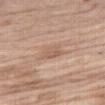<case>
<biopsy_status>not biopsied; imaged during a skin examination</biopsy_status>
<image>
  <source>total-body photography crop</source>
  <field_of_view_mm>15</field_of_view_mm>
</image>
<lighting>white-light</lighting>
<site>right thigh</site>
<automated_metrics>
  <area_mm2_approx>4.5</area_mm2_approx>
  <eccentricity>0.6</eccentricity>
  <shape_asymmetry>0.25</shape_asymmetry>
  <cielab_L>59</cielab_L>
  <cielab_a>19</cielab_a>
  <cielab_b>29</cielab_b>
  <vs_skin_darker_L>7.0</vs_skin_darker_L>
  <vs_skin_contrast_norm>5.0</vs_skin_contrast_norm>
  <border_irregularity_0_10>2.0</border_irregularity_0_10>
  <color_variation_0_10>2.0</color_variation_0_10>
  <peripheral_color_asymmetry>0.5</peripheral_color_asymmetry>
</automated_metrics>
<patient>
  <sex>male</sex>
  <age_approx>70</age_approx>
</patient>
<lesion_size>
  <long_diameter_mm_approx>2.5</long_diameter_mm_approx>
</lesion_size>
</case>